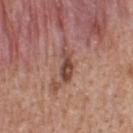Case summary:
– follow-up — total-body-photography surveillance lesion; no biopsy
– image source — ~15 mm tile from a whole-body skin photo
– size — ≈3.5 mm
– anatomic site — the upper back
– automated lesion analysis — a lesion area of about 5 mm² and an outline eccentricity of about 0.9 (0 = round, 1 = elongated); a nevus-likeness score of about 35/100
– subject — male, approximately 40 years of age